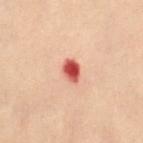No biopsy was performed on this lesion — it was imaged during a full skin examination and was not determined to be concerning.